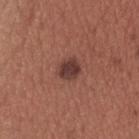<tbp_lesion>
  <biopsy_status>not biopsied; imaged during a skin examination</biopsy_status>
  <site>head or neck</site>
  <lesion_size>
    <long_diameter_mm_approx>2.5</long_diameter_mm_approx>
  </lesion_size>
  <patient>
    <sex>male</sex>
    <age_approx>35</age_approx>
  </patient>
  <lighting>white-light</lighting>
  <image>
    <source>total-body photography crop</source>
    <field_of_view_mm>15</field_of_view_mm>
  </image>
</tbp_lesion>